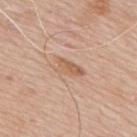  image:
    source: total-body photography crop
    field_of_view_mm: 15
  site: back
  lighting: white-light
  patient:
    sex: male
    age_approx: 65
  lesion_size:
    long_diameter_mm_approx: 3.5
  automated_metrics:
    area_mm2_approx: 5.0
    shape_asymmetry: 0.4
    border_irregularity_0_10: 4.0
    color_variation_0_10: 2.5
    peripheral_color_asymmetry: 1.0
    nevus_likeness_0_100: 0
    lesion_detection_confidence_0_100: 100